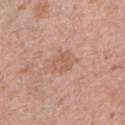Captured during whole-body skin photography for melanoma surveillance; the lesion was not biopsied. The lesion is located on the right upper arm. A roughly 15 mm field-of-view crop from a total-body skin photograph. This is a white-light tile. The subject is a female roughly 40 years of age.